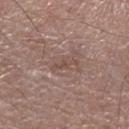Q: Was this lesion biopsied?
A: no biopsy performed (imaged during a skin exam)
Q: Lesion location?
A: the left forearm
Q: What is the imaging modality?
A: ~15 mm crop, total-body skin-cancer survey
Q: Patient demographics?
A: male, aged 63 to 67